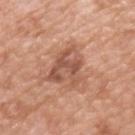Imaged during a routine full-body skin examination; the lesion was not biopsied and no histopathology is available.